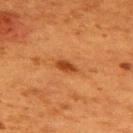This lesion was catalogued during total-body skin photography and was not selected for biopsy. The lesion's longest dimension is about 3 mm. A 15 mm crop from a total-body photograph taken for skin-cancer surveillance. The lesion is on the upper back. Imaged with cross-polarized lighting. A female patient roughly 50 years of age.